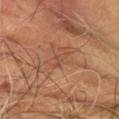Clinical impression: This lesion was catalogued during total-body skin photography and was not selected for biopsy. Acquisition and patient details: The lesion is located on the left arm. The subject is a male in their 60s. A 15 mm close-up tile from a total-body photography series done for melanoma screening.